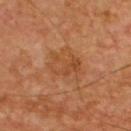workup = total-body-photography surveillance lesion; no biopsy | lesion size = ≈3.5 mm | illumination = cross-polarized | subject = male, roughly 55 years of age | image = total-body-photography crop, ~15 mm field of view | site = the chest.